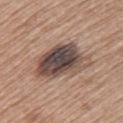Clinical impression:
Captured during whole-body skin photography for melanoma surveillance; the lesion was not biopsied.
Image and clinical context:
A 15 mm crop from a total-body photograph taken for skin-cancer surveillance. A female subject, aged 58 to 62. Automated image analysis of the tile measured a footprint of about 21 mm², an eccentricity of roughly 0.8, and a symmetry-axis asymmetry near 0.3. And it measured a classifier nevus-likeness of about 5/100. Approximately 7 mm at its widest. The lesion is on the back. Captured under white-light illumination.